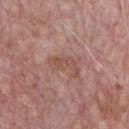workup = no biopsy performed (imaged during a skin exam) | image-analysis metrics = an average lesion color of about L≈51 a*≈21 b*≈26 (CIELAB) and a normalized border contrast of about 6; border irregularity of about 7 on a 0–10 scale, internal color variation of about 1.5 on a 0–10 scale, and radial color variation of about 0.5 | lesion size = ~4 mm (longest diameter) | site = the chest | image source = ~15 mm tile from a whole-body skin photo | subject = male, aged 68–72 | illumination = white-light.